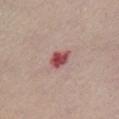No biopsy was performed on this lesion — it was imaged during a full skin examination and was not determined to be concerning. Cropped from a whole-body photographic skin survey; the tile spans about 15 mm. A female subject, roughly 65 years of age. Located on the chest.